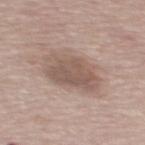Case summary:
* biopsy status — total-body-photography surveillance lesion; no biopsy
* image — ~15 mm tile from a whole-body skin photo
* subject — male, in their mid- to late 70s
* image-analysis metrics — a footprint of about 17 mm², an eccentricity of roughly 0.7, and two-axis asymmetry of about 0.25; a mean CIELAB color near L≈55 a*≈15 b*≈24, about 10 CIELAB-L* units darker than the surrounding skin, and a lesion-to-skin contrast of about 7 (normalized; higher = more distinct); border irregularity of about 2.5 on a 0–10 scale and internal color variation of about 3.5 on a 0–10 scale
* diameter — ≈5.5 mm
* anatomic site — the mid back